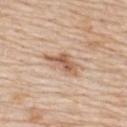Recorded during total-body skin imaging; not selected for excision or biopsy. A 15 mm crop from a total-body photograph taken for skin-cancer surveillance. The tile uses white-light illumination. Longest diameter approximately 4.5 mm. The patient is a female aged 68–72. Automated tile analysis of the lesion measured a lesion color around L≈60 a*≈19 b*≈32 in CIELAB and a normalized lesion–skin contrast near 8. The software also gave border irregularity of about 4 on a 0–10 scale, internal color variation of about 4.5 on a 0–10 scale, and a peripheral color-asymmetry measure near 1.5. It also reported a lesion-detection confidence of about 100/100. The lesion is on the back.